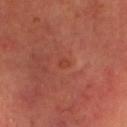Assessment:
Part of a total-body skin-imaging series; this lesion was reviewed on a skin check and was not flagged for biopsy.